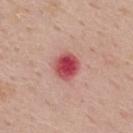| field | value |
|---|---|
| biopsy status | catalogued during a skin exam; not biopsied |
| subject | male, aged 53–57 |
| imaging modality | ~15 mm crop, total-body skin-cancer survey |
| lighting | white-light |
| automated lesion analysis | two-axis asymmetry of about 0.1; an automated nevus-likeness rating near 0 out of 100 and a lesion-detection confidence of about 100/100 |
| lesion diameter | ≈3 mm |
| anatomic site | the upper back |